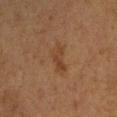<record>
<biopsy_status>not biopsied; imaged during a skin examination</biopsy_status>
<lighting>cross-polarized</lighting>
<automated_metrics>
  <cielab_L>35</cielab_L>
  <cielab_a>17</cielab_a>
  <cielab_b>28</cielab_b>
  <vs_skin_contrast_norm>5.5</vs_skin_contrast_norm>
</automated_metrics>
<patient>
  <sex>female</sex>
  <age_approx>40</age_approx>
</patient>
<lesion_size>
  <long_diameter_mm_approx>3.5</long_diameter_mm_approx>
</lesion_size>
<site>arm</site>
<image>
  <source>total-body photography crop</source>
  <field_of_view_mm>15</field_of_view_mm>
</image>
</record>